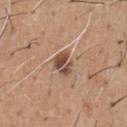- biopsy status · catalogued during a skin exam; not biopsied